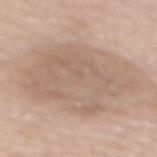Q: Was a biopsy performed?
A: imaged on a skin check; not biopsied
Q: What kind of image is this?
A: total-body-photography crop, ~15 mm field of view
Q: Lesion location?
A: the mid back
Q: Automated lesion metrics?
A: an average lesion color of about L≈62 a*≈15 b*≈26 (CIELAB), about 8 CIELAB-L* units darker than the surrounding skin, and a normalized lesion–skin contrast near 5.5
Q: Lesion size?
A: ≈10 mm
Q: What are the patient's age and sex?
A: female, roughly 60 years of age